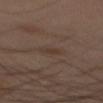| key | value |
|---|---|
| biopsy status | catalogued during a skin exam; not biopsied |
| lesion size | about 2.5 mm |
| image | total-body-photography crop, ~15 mm field of view |
| anatomic site | the mid back |
| patient | male, aged approximately 50 |